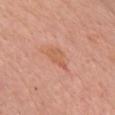Captured during whole-body skin photography for melanoma surveillance; the lesion was not biopsied.